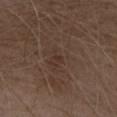Q: Where on the body is the lesion?
A: the abdomen
Q: What are the patient's age and sex?
A: male, aged 68–72
Q: What kind of image is this?
A: ~15 mm tile from a whole-body skin photo
Q: What lighting was used for the tile?
A: white-light
Q: What is the lesion's diameter?
A: about 1 mm
Q: Automated lesion metrics?
A: a mean CIELAB color near L≈31 a*≈16 b*≈24, a lesion–skin lightness drop of about 5, and a lesion-to-skin contrast of about 5 (normalized; higher = more distinct); an automated nevus-likeness rating near 0 out of 100 and a lesion-detection confidence of about 100/100